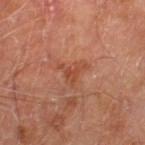workup = imaged on a skin check; not biopsied | site = the left thigh | patient = male, aged 68 to 72 | image source = total-body-photography crop, ~15 mm field of view.